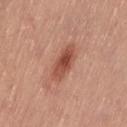Clinical impression:
The lesion was photographed on a routine skin check and not biopsied; there is no pathology result.
Context:
A 15 mm crop from a total-body photograph taken for skin-cancer surveillance. A female subject roughly 55 years of age. From the left thigh.